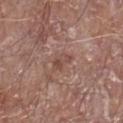notes = imaged on a skin check; not biopsied | imaging modality = ~15 mm tile from a whole-body skin photo | patient = male, in their 70s | anatomic site = the right lower leg | lesion diameter = ≈3 mm | lighting = white-light illumination.